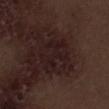<record>
  <biopsy_status>not biopsied; imaged during a skin examination</biopsy_status>
  <lighting>white-light</lighting>
  <patient>
    <sex>male</sex>
    <age_approx>70</age_approx>
  </patient>
  <site>right thigh</site>
  <image>
    <source>total-body photography crop</source>
    <field_of_view_mm>15</field_of_view_mm>
  </image>
  <lesion_size>
    <long_diameter_mm_approx>4.5</long_diameter_mm_approx>
  </lesion_size>
</record>